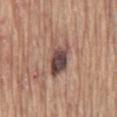Q: Was this lesion biopsied?
A: catalogued during a skin exam; not biopsied
Q: Illumination type?
A: white-light illumination
Q: What is the lesion's diameter?
A: ≈5 mm
Q: Lesion location?
A: the mid back
Q: How was this image acquired?
A: total-body-photography crop, ~15 mm field of view
Q: What are the patient's age and sex?
A: male, approximately 65 years of age